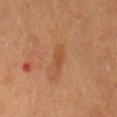Impression:
The lesion was photographed on a routine skin check and not biopsied; there is no pathology result.
Image and clinical context:
The patient is a male approximately 60 years of age. A 15 mm close-up tile from a total-body photography series done for melanoma screening. The tile uses cross-polarized illumination. Measured at roughly 3 mm in maximum diameter. Located on the abdomen.